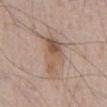On the chest. Captured under white-light illumination. Automated image analysis of the tile measured a border-irregularity index near 4.5/10, internal color variation of about 8.5 on a 0–10 scale, and peripheral color asymmetry of about 3. The analysis additionally found an automated nevus-likeness rating near 55 out of 100 and lesion-presence confidence of about 100/100. Longest diameter approximately 5 mm. A region of skin cropped from a whole-body photographic capture, roughly 15 mm wide. The subject is a male aged around 65.